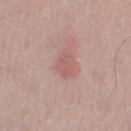  biopsy_status: not biopsied; imaged during a skin examination
  patient:
    sex: male
    age_approx: 65
  lighting: white-light
  image:
    source: total-body photography crop
    field_of_view_mm: 15
  site: left upper arm
  automated_metrics:
    area_mm2_approx: 5.0
    eccentricity: 0.75
    shape_asymmetry: 0.2
    cielab_L: 58
    cielab_a: 24
    cielab_b: 23
    vs_skin_darker_L: 7.0
    vs_skin_contrast_norm: 5.0
    nevus_likeness_0_100: 25
    lesion_detection_confidence_0_100: 100